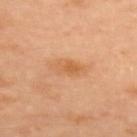Q: Is there a histopathology result?
A: catalogued during a skin exam; not biopsied
Q: How was the tile lit?
A: cross-polarized
Q: How was this image acquired?
A: ~15 mm crop, total-body skin-cancer survey
Q: What is the anatomic site?
A: the upper back